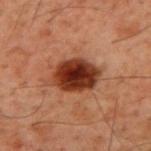Impression:
The lesion was photographed on a routine skin check and not biopsied; there is no pathology result.
Background:
Captured under cross-polarized illumination. The total-body-photography lesion software estimated a shape-asymmetry score of about 0.15 (0 = symmetric). The analysis additionally found an average lesion color of about L≈26 a*≈22 b*≈26 (CIELAB) and roughly 15 lightness units darker than nearby skin. The lesion is located on the upper back. A 15 mm crop from a total-body photograph taken for skin-cancer surveillance. A male patient roughly 60 years of age.